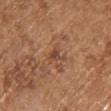Recorded during total-body skin imaging; not selected for excision or biopsy.
Approximately 3 mm at its widest.
The subject is a male approximately 70 years of age.
The lesion is located on the upper back.
A roughly 15 mm field-of-view crop from a total-body skin photograph.
The tile uses white-light illumination.